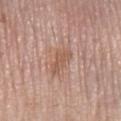No biopsy was performed on this lesion — it was imaged during a full skin examination and was not determined to be concerning. Automated tile analysis of the lesion measured a footprint of about 5.5 mm² and a symmetry-axis asymmetry near 0.45. The analysis additionally found border irregularity of about 4.5 on a 0–10 scale, a within-lesion color-variation index near 1.5/10, and radial color variation of about 0.5. The lesion is located on the right lower leg. A roughly 15 mm field-of-view crop from a total-body skin photograph. The subject is a female about 65 years old. This is a white-light tile. Longest diameter approximately 3.5 mm.